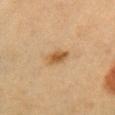biopsy status: total-body-photography surveillance lesion; no biopsy
anatomic site: the chest
automated lesion analysis: a lesion area of about 5 mm², an eccentricity of roughly 0.8, and a shape-asymmetry score of about 0.2 (0 = symmetric)
tile lighting: cross-polarized
lesion diameter: about 3 mm
imaging modality: total-body-photography crop, ~15 mm field of view
subject: female, aged around 30